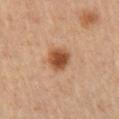Clinical impression: Captured during whole-body skin photography for melanoma surveillance; the lesion was not biopsied. Clinical summary: The lesion is located on the right upper arm. This is a cross-polarized tile. A female subject, in their mid- to late 40s. The lesion's longest dimension is about 3.5 mm. A region of skin cropped from a whole-body photographic capture, roughly 15 mm wide.